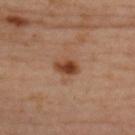The lesion was photographed on a routine skin check and not biopsied; there is no pathology result.
Longest diameter approximately 3 mm.
The lesion is located on the back.
Automated tile analysis of the lesion measured a lesion area of about 4.5 mm², an eccentricity of roughly 0.8, and a symmetry-axis asymmetry near 0.25. The analysis additionally found a mean CIELAB color near L≈42 a*≈24 b*≈33, about 13 CIELAB-L* units darker than the surrounding skin, and a normalized lesion–skin contrast near 10. It also reported an automated nevus-likeness rating near 95 out of 100 and a lesion-detection confidence of about 100/100.
A 15 mm close-up extracted from a 3D total-body photography capture.
The subject is a female roughly 40 years of age.